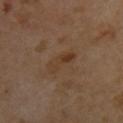The lesion was photographed on a routine skin check and not biopsied; there is no pathology result. Approximately 4 mm at its widest. The tile uses cross-polarized illumination. From the upper back. A female subject, in their 40s. A region of skin cropped from a whole-body photographic capture, roughly 15 mm wide.